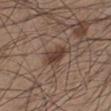Clinical impression: Recorded during total-body skin imaging; not selected for excision or biopsy.